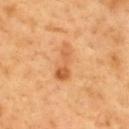  biopsy_status: not biopsied; imaged during a skin examination
  automated_metrics:
    area_mm2_approx: 6.0
    shape_asymmetry: 0.45
    nevus_likeness_0_100: 20
    lesion_detection_confidence_0_100: 100
  patient:
    sex: male
    age_approx: 50
  lesion_size:
    long_diameter_mm_approx: 4.5
  image:
    source: total-body photography crop
    field_of_view_mm: 15
  lighting: cross-polarized
  site: mid back